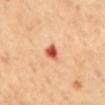workup: catalogued during a skin exam; not biopsied
TBP lesion metrics: a footprint of about 4 mm² and an eccentricity of roughly 0.65; a mean CIELAB color near L≈61 a*≈36 b*≈38, about 17 CIELAB-L* units darker than the surrounding skin, and a normalized lesion–skin contrast near 10; a border-irregularity rating of about 3/10, a color-variation rating of about 6/10, and radial color variation of about 1.5
lighting: cross-polarized illumination
location: the back
imaging modality: total-body-photography crop, ~15 mm field of view
patient: female, in their mid- to late 50s